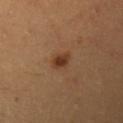notes: no biopsy performed (imaged during a skin exam); patient: female, in their mid-30s; anatomic site: the left upper arm; image source: 15 mm crop, total-body photography; tile lighting: cross-polarized; automated lesion analysis: an area of roughly 4 mm², an outline eccentricity of about 0.65 (0 = round, 1 = elongated), and a symmetry-axis asymmetry near 0.2; diameter: about 2.5 mm.